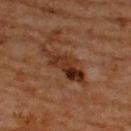Q: Was this lesion biopsied?
A: imaged on a skin check; not biopsied
Q: What is the imaging modality?
A: 15 mm crop, total-body photography
Q: Where on the body is the lesion?
A: the upper back
Q: Patient demographics?
A: male, about 65 years old